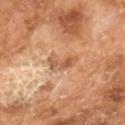notes: no biopsy performed (imaged during a skin exam); acquisition: ~15 mm crop, total-body skin-cancer survey; patient: male, aged around 65; lighting: cross-polarized illumination; size: ≈4 mm.